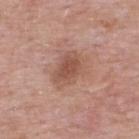Case summary:
• follow-up · imaged on a skin check; not biopsied
• location · the upper back
• lighting · white-light illumination
• subject · male, aged approximately 55
• acquisition · 15 mm crop, total-body photography
• size · ≈4.5 mm